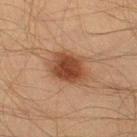This lesion was catalogued during total-body skin photography and was not selected for biopsy. The lesion is located on the right thigh. A 15 mm close-up extracted from a 3D total-body photography capture. A male patient, roughly 50 years of age. Automated image analysis of the tile measured a footprint of about 13 mm² and two-axis asymmetry of about 0.2. And it measured a lesion color around L≈36 a*≈19 b*≈27 in CIELAB. And it measured an automated nevus-likeness rating near 100 out of 100 and a lesion-detection confidence of about 100/100. The tile uses cross-polarized illumination.